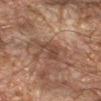Part of a total-body skin-imaging series; this lesion was reviewed on a skin check and was not flagged for biopsy.
A male subject approximately 65 years of age.
The lesion's longest dimension is about 3.5 mm.
A 15 mm crop from a total-body photograph taken for skin-cancer surveillance.
The lesion-visualizer software estimated a footprint of about 5.5 mm², an eccentricity of roughly 0.85, and a shape-asymmetry score of about 0.35 (0 = symmetric). The software also gave an average lesion color of about L≈34 a*≈15 b*≈22 (CIELAB), roughly 6 lightness units darker than nearby skin, and a lesion-to-skin contrast of about 6 (normalized; higher = more distinct). The analysis additionally found an automated nevus-likeness rating near 5 out of 100 and lesion-presence confidence of about 95/100.
The lesion is on the right forearm.